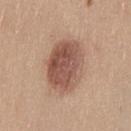| key | value |
|---|---|
| notes | no biopsy performed (imaged during a skin exam) |
| site | the leg |
| illumination | white-light illumination |
| subject | female, aged 38–42 |
| image | 15 mm crop, total-body photography |
| lesion diameter | ≈6 mm |